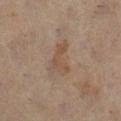Clinical impression: Captured during whole-body skin photography for melanoma surveillance; the lesion was not biopsied. Background: Located on the right lower leg. The tile uses cross-polarized illumination. A female patient in their 50s. Measured at roughly 4 mm in maximum diameter. Automated image analysis of the tile measured an average lesion color of about L≈48 a*≈15 b*≈27 (CIELAB), roughly 6 lightness units darker than nearby skin, and a lesion-to-skin contrast of about 6 (normalized; higher = more distinct). The software also gave border irregularity of about 6 on a 0–10 scale and internal color variation of about 2 on a 0–10 scale. A region of skin cropped from a whole-body photographic capture, roughly 15 mm wide.